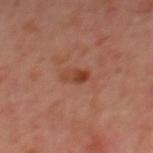<case>
<image>
  <source>total-body photography crop</source>
  <field_of_view_mm>15</field_of_view_mm>
</image>
<patient>
  <sex>male</sex>
  <age_approx>65</age_approx>
</patient>
<site>mid back</site>
<lighting>cross-polarized</lighting>
<automated_metrics>
  <area_mm2_approx>4.0</area_mm2_approx>
  <eccentricity>0.85</eccentricity>
  <shape_asymmetry>0.3</shape_asymmetry>
  <color_variation_0_10>6.5</color_variation_0_10>
  <peripheral_color_asymmetry>2.5</peripheral_color_asymmetry>
  <lesion_detection_confidence_0_100>100</lesion_detection_confidence_0_100>
</automated_metrics>
</case>